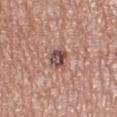Recorded during total-body skin imaging; not selected for excision or biopsy. The lesion's longest dimension is about 3 mm. The subject is a female roughly 60 years of age. The lesion is on the left lower leg. Cropped from a whole-body photographic skin survey; the tile spans about 15 mm.